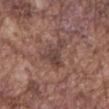Impression:
Recorded during total-body skin imaging; not selected for excision or biopsy.
Clinical summary:
Automated tile analysis of the lesion measured border irregularity of about 7 on a 0–10 scale, a within-lesion color-variation index near 3/10, and peripheral color asymmetry of about 1. The analysis additionally found a detector confidence of about 95 out of 100 that the crop contains a lesion. A male patient aged around 75. This image is a 15 mm lesion crop taken from a total-body photograph. This is a white-light tile. The lesion is on the mid back. Measured at roughly 5 mm in maximum diameter.